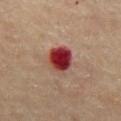Assessment: This lesion was catalogued during total-body skin photography and was not selected for biopsy. Clinical summary: The lesion is on the front of the torso. A male subject, in their mid- to late 80s or older. Automated image analysis of the tile measured a lesion color around L≈37 a*≈33 b*≈25 in CIELAB and a normalized border contrast of about 14.5. The analysis additionally found a border-irregularity index near 1.5/10, a color-variation rating of about 8.5/10, and peripheral color asymmetry of about 2.5. Cropped from a whole-body photographic skin survey; the tile spans about 15 mm. Captured under cross-polarized illumination.